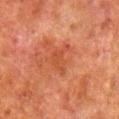biopsy status = imaged on a skin check; not biopsied
lighting = cross-polarized
size = ~3.5 mm (longest diameter)
subject = male, aged 78–82
acquisition = 15 mm crop, total-body photography
automated lesion analysis = a lesion color around L≈40 a*≈27 b*≈32 in CIELAB, a lesion–skin lightness drop of about 5, and a normalized lesion–skin contrast near 5; a classifier nevus-likeness of about 0/100 and a detector confidence of about 100 out of 100 that the crop contains a lesion
body site = the right lower leg